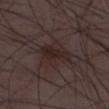Imaged during a routine full-body skin examination; the lesion was not biopsied and no histopathology is available. A 15 mm close-up extracted from a 3D total-body photography capture. A male patient aged around 50.